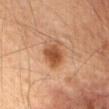Impression: Part of a total-body skin-imaging series; this lesion was reviewed on a skin check and was not flagged for biopsy. Clinical summary: The lesion is located on the chest. A 15 mm close-up extracted from a 3D total-body photography capture. The subject is a male aged approximately 85 or older. Imaged with cross-polarized lighting.